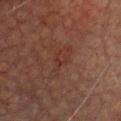| field | value |
|---|---|
| workup | catalogued during a skin exam; not biopsied |
| image | 15 mm crop, total-body photography |
| location | the chest |
| size | ~5 mm (longest diameter) |
| subject | male, about 75 years old |